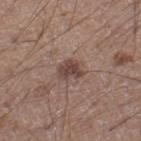Recorded during total-body skin imaging; not selected for excision or biopsy.
A roughly 15 mm field-of-view crop from a total-body skin photograph.
The subject is a male about 20 years old.
This is a white-light tile.
The lesion is located on the right lower leg.